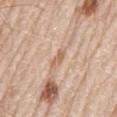Impression: Recorded during total-body skin imaging; not selected for excision or biopsy.